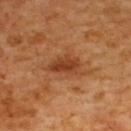Findings:
- notes · no biopsy performed (imaged during a skin exam)
- subject · male, approximately 60 years of age
- image source · total-body-photography crop, ~15 mm field of view
- location · the upper back